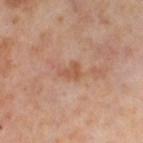  biopsy_status: not biopsied; imaged during a skin examination
  patient:
    sex: female
    age_approx: 55
  site: left thigh
  image:
    source: total-body photography crop
    field_of_view_mm: 15